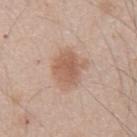Assessment: The lesion was tiled from a total-body skin photograph and was not biopsied. Context: The recorded lesion diameter is about 4 mm. A male patient, approximately 45 years of age. A 15 mm close-up tile from a total-body photography series done for melanoma screening. Automated image analysis of the tile measured an area of roughly 11 mm² and an eccentricity of roughly 0.5. On the abdomen.